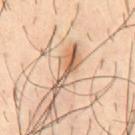{"biopsy_status": "not biopsied; imaged during a skin examination", "automated_metrics": {"border_irregularity_0_10": 5.5, "color_variation_0_10": 9.0, "lesion_detection_confidence_0_100": 60}, "lesion_size": {"long_diameter_mm_approx": 6.0}, "lighting": "cross-polarized", "site": "chest", "image": {"source": "total-body photography crop", "field_of_view_mm": 15}, "patient": {"sex": "male", "age_approx": 40}}